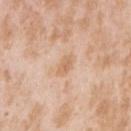Case summary:
– follow-up: imaged on a skin check; not biopsied
– body site: the right upper arm
– image-analysis metrics: a lesion area of about 3.5 mm², a shape eccentricity near 0.8, and two-axis asymmetry of about 0.25; a mean CIELAB color near L≈66 a*≈19 b*≈35, a lesion–skin lightness drop of about 8, and a normalized lesion–skin contrast near 6; internal color variation of about 2 on a 0–10 scale and a peripheral color-asymmetry measure near 0.5
– tile lighting: white-light illumination
– acquisition: 15 mm crop, total-body photography
– patient: female, about 25 years old